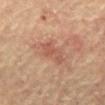notes = total-body-photography surveillance lesion; no biopsy | body site = the abdomen | illumination = cross-polarized illumination | lesion diameter = ≈3.5 mm | patient = male, in their mid-60s | image = ~15 mm tile from a whole-body skin photo.